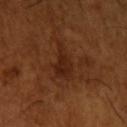- notes — no biopsy performed (imaged during a skin exam)
- tile lighting — cross-polarized
- image — total-body-photography crop, ~15 mm field of view
- location — the left upper arm
- subject — male, in their mid-60s